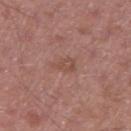  biopsy_status: not biopsied; imaged during a skin examination
  automated_metrics:
    area_mm2_approx: 3.5
    eccentricity: 0.8
    vs_skin_darker_L: 6.0
    border_irregularity_0_10: 4.0
    color_variation_0_10: 2.0
    peripheral_color_asymmetry: 1.0
  site: left thigh
  image:
    source: total-body photography crop
    field_of_view_mm: 15
  lighting: white-light
  patient:
    sex: male
    age_approx: 55
  lesion_size:
    long_diameter_mm_approx: 3.0